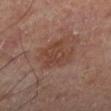Recorded during total-body skin imaging; not selected for excision or biopsy. Cropped from a total-body skin-imaging series; the visible field is about 15 mm. Longest diameter approximately 4 mm. The patient is a male aged 63 to 67. The lesion is on the left lower leg. The tile uses cross-polarized illumination.